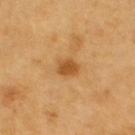Impression:
Captured during whole-body skin photography for melanoma surveillance; the lesion was not biopsied.
Acquisition and patient details:
The subject is a male aged approximately 60. Captured under cross-polarized illumination. About 2.5 mm across. Cropped from a whole-body photographic skin survey; the tile spans about 15 mm. From the upper back.